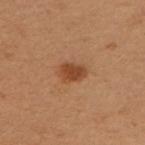Assessment: Part of a total-body skin-imaging series; this lesion was reviewed on a skin check and was not flagged for biopsy. Image and clinical context: On the arm. This image is a 15 mm lesion crop taken from a total-body photograph. The patient is a female roughly 50 years of age. Approximately 3 mm at its widest. The tile uses cross-polarized illumination. The lesion-visualizer software estimated two-axis asymmetry of about 0.15. The software also gave an automated nevus-likeness rating near 95 out of 100 and a lesion-detection confidence of about 100/100.